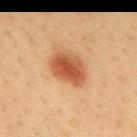<lesion>
  <lighting>cross-polarized</lighting>
  <automated_metrics>
    <area_mm2_approx>12.0</area_mm2_approx>
    <eccentricity>0.75</eccentricity>
    <border_irregularity_0_10>1.5</border_irregularity_0_10>
    <color_variation_0_10>5.0</color_variation_0_10>
    <peripheral_color_asymmetry>1.5</peripheral_color_asymmetry>
    <nevus_likeness_0_100>100</nevus_likeness_0_100>
    <lesion_detection_confidence_0_100>100</lesion_detection_confidence_0_100>
  </automated_metrics>
  <patient>
    <sex>female</sex>
    <age_approx>40</age_approx>
  </patient>
  <site>mid back</site>
  <image>
    <source>total-body photography crop</source>
    <field_of_view_mm>15</field_of_view_mm>
  </image>
</lesion>